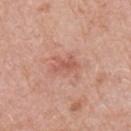Longest diameter approximately 3 mm. The lesion-visualizer software estimated an area of roughly 4 mm², an eccentricity of roughly 0.85, and a symmetry-axis asymmetry near 0.4. It also reported an automated nevus-likeness rating near 0 out of 100 and a detector confidence of about 100 out of 100 that the crop contains a lesion. A lesion tile, about 15 mm wide, cut from a 3D total-body photograph. The tile uses white-light illumination. From the left arm. The patient is a male roughly 80 years of age.